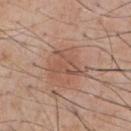The lesion was photographed on a routine skin check and not biopsied; there is no pathology result. Cropped from a total-body skin-imaging series; the visible field is about 15 mm. Captured under white-light illumination. Located on the front of the torso. The patient is a male aged approximately 60. The total-body-photography lesion software estimated a lesion color around L≈52 a*≈21 b*≈28 in CIELAB, roughly 8 lightness units darker than nearby skin, and a lesion-to-skin contrast of about 5.5 (normalized; higher = more distinct). The analysis additionally found an automated nevus-likeness rating near 0 out of 100. The lesion's longest dimension is about 4 mm.